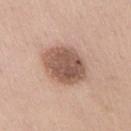Captured during whole-body skin photography for melanoma surveillance; the lesion was not biopsied. The subject is a male roughly 40 years of age. A roughly 15 mm field-of-view crop from a total-body skin photograph. The lesion's longest dimension is about 6 mm. This is a white-light tile. An algorithmic analysis of the crop reported a mean CIELAB color near L≈55 a*≈19 b*≈27, roughly 14 lightness units darker than nearby skin, and a lesion-to-skin contrast of about 9.5 (normalized; higher = more distinct). The analysis additionally found an automated nevus-likeness rating near 20 out of 100 and lesion-presence confidence of about 100/100. From the chest.